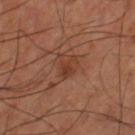body site: the leg
image: ~15 mm tile from a whole-body skin photo
size: ~2.5 mm (longest diameter)
lighting: cross-polarized
patient: male, approximately 60 years of age